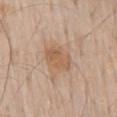Part of a total-body skin-imaging series; this lesion was reviewed on a skin check and was not flagged for biopsy.
The patient is a male about 60 years old.
The recorded lesion diameter is about 4 mm.
The lesion is on the mid back.
The tile uses white-light illumination.
A 15 mm close-up tile from a total-body photography series done for melanoma screening.
Automated tile analysis of the lesion measured an area of roughly 7.5 mm² and two-axis asymmetry of about 0.2. It also reported an average lesion color of about L≈58 a*≈19 b*≈33 (CIELAB), about 8 CIELAB-L* units darker than the surrounding skin, and a normalized lesion–skin contrast near 6.